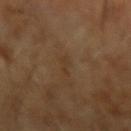Clinical impression:
The lesion was tiled from a total-body skin photograph and was not biopsied.
Image and clinical context:
A male subject aged approximately 65. Longest diameter approximately 3 mm. An algorithmic analysis of the crop reported roughly 4 lightness units darker than nearby skin and a lesion-to-skin contrast of about 4.5 (normalized; higher = more distinct). It also reported a border-irregularity index near 6/10. It also reported an automated nevus-likeness rating near 0 out of 100 and lesion-presence confidence of about 85/100. This image is a 15 mm lesion crop taken from a total-body photograph.